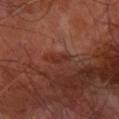This lesion was catalogued during total-body skin photography and was not selected for biopsy.
On the arm.
A male patient in their mid- to late 60s.
Approximately 2.5 mm at its widest.
A close-up tile cropped from a whole-body skin photograph, about 15 mm across.
The tile uses cross-polarized illumination.
Automated image analysis of the tile measured border irregularity of about 3.5 on a 0–10 scale and a color-variation rating of about 0/10. The analysis additionally found a classifier nevus-likeness of about 15/100 and lesion-presence confidence of about 100/100.